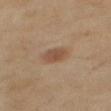Q: Was a biopsy performed?
A: total-body-photography surveillance lesion; no biopsy
Q: Illumination type?
A: cross-polarized illumination
Q: Patient demographics?
A: female, aged 58 to 62
Q: How was this image acquired?
A: total-body-photography crop, ~15 mm field of view
Q: Where on the body is the lesion?
A: the right thigh